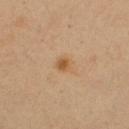  biopsy_status: not biopsied; imaged during a skin examination
  image:
    source: total-body photography crop
    field_of_view_mm: 15
  site: left upper arm
  patient:
    sex: female
    age_approx: 40